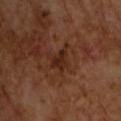<tbp_lesion>
<biopsy_status>not biopsied; imaged during a skin examination</biopsy_status>
<lighting>cross-polarized</lighting>
<patient>
  <sex>male</sex>
  <age_approx>65</age_approx>
</patient>
<lesion_size>
  <long_diameter_mm_approx>2.5</long_diameter_mm_approx>
</lesion_size>
<image>
  <source>total-body photography crop</source>
  <field_of_view_mm>15</field_of_view_mm>
</image>
<automated_metrics>
  <area_mm2_approx>4.5</area_mm2_approx>
  <eccentricity>0.55</eccentricity>
  <shape_asymmetry>0.45</shape_asymmetry>
  <border_irregularity_0_10>4.0</border_irregularity_0_10>
  <color_variation_0_10>2.0</color_variation_0_10>
  <peripheral_color_asymmetry>0.5</peripheral_color_asymmetry>
  <nevus_likeness_0_100>0</nevus_likeness_0_100>
  <lesion_detection_confidence_0_100>100</lesion_detection_confidence_0_100>
</automated_metrics>
</tbp_lesion>